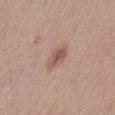The lesion was photographed on a routine skin check and not biopsied; there is no pathology result.
The lesion is on the left thigh.
A female subject approximately 40 years of age.
A region of skin cropped from a whole-body photographic capture, roughly 15 mm wide.